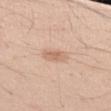The lesion was tiled from a total-body skin photograph and was not biopsied.
This image is a 15 mm lesion crop taken from a total-body photograph.
Located on the left upper arm.
A female subject, in their mid- to late 20s.
Measured at roughly 3 mm in maximum diameter.
This is a white-light tile.
An algorithmic analysis of the crop reported a lesion area of about 5 mm², an eccentricity of roughly 0.75, and two-axis asymmetry of about 0.25. It also reported a border-irregularity rating of about 2/10.